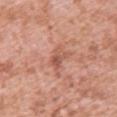  biopsy_status: not biopsied; imaged during a skin examination
  image:
    source: total-body photography crop
    field_of_view_mm: 15
  lesion_size:
    long_diameter_mm_approx: 3.0
  patient:
    sex: female
    age_approx: 40
  site: upper back
  automated_metrics:
    area_mm2_approx: 4.5
    eccentricity: 0.85
    shape_asymmetry: 0.35
    cielab_L: 56
    cielab_a: 25
    cielab_b: 30
    vs_skin_darker_L: 9.0
    vs_skin_contrast_norm: 6.0
    nevus_likeness_0_100: 0
  lighting: white-light